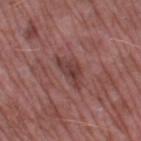Impression:
This lesion was catalogued during total-body skin photography and was not selected for biopsy.
Image and clinical context:
The lesion is located on the left thigh. Cropped from a total-body skin-imaging series; the visible field is about 15 mm. Captured under white-light illumination. The lesion's longest dimension is about 4.5 mm. An algorithmic analysis of the crop reported a shape eccentricity near 0.85 and a symmetry-axis asymmetry near 0.45. The analysis additionally found an average lesion color of about L≈40 a*≈23 b*≈21 (CIELAB), roughly 8 lightness units darker than nearby skin, and a normalized border contrast of about 7. The analysis additionally found border irregularity of about 4.5 on a 0–10 scale and a peripheral color-asymmetry measure near 1.5. A male subject, aged around 55.